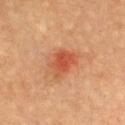Case summary:
- workup — no biopsy performed (imaged during a skin exam)
- imaging modality — 15 mm crop, total-body photography
- patient — female, aged around 60
- body site — the chest